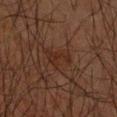This lesion was catalogued during total-body skin photography and was not selected for biopsy. On the right upper arm. A 15 mm close-up extracted from a 3D total-body photography capture. A male subject aged 63–67.